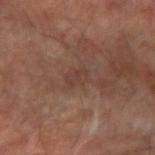Clinical impression:
No biopsy was performed on this lesion — it was imaged during a full skin examination and was not determined to be concerning.
Acquisition and patient details:
The lesion is located on the right forearm. Cropped from a total-body skin-imaging series; the visible field is about 15 mm. Automated image analysis of the tile measured an area of roughly 4 mm², an outline eccentricity of about 0.7 (0 = round, 1 = elongated), and a symmetry-axis asymmetry near 0.2. Imaged with cross-polarized lighting.